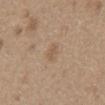workup — total-body-photography surveillance lesion; no biopsy | image — ~15 mm tile from a whole-body skin photo | site — the front of the torso | lesion diameter — ~2.5 mm (longest diameter) | patient — male, aged 63–67 | tile lighting — white-light.